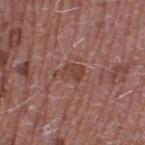Findings:
- workup · total-body-photography surveillance lesion; no biopsy
- lesion diameter · ~3 mm (longest diameter)
- illumination · white-light
- TBP lesion metrics · an area of roughly 4 mm², a shape eccentricity near 0.8, and a symmetry-axis asymmetry near 0.35; an average lesion color of about L≈42 a*≈22 b*≈24 (CIELAB), about 7 CIELAB-L* units darker than the surrounding skin, and a normalized border contrast of about 6; internal color variation of about 1.5 on a 0–10 scale and a peripheral color-asymmetry measure near 0.5
- imaging modality · 15 mm crop, total-body photography
- subject · male, approximately 60 years of age
- anatomic site · the leg